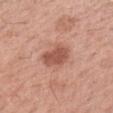Assessment: The lesion was tiled from a total-body skin photograph and was not biopsied. Acquisition and patient details: A roughly 15 mm field-of-view crop from a total-body skin photograph. The lesion-visualizer software estimated an area of roughly 8 mm² and two-axis asymmetry of about 0.25. Imaged with white-light lighting. A female subject, in their mid- to late 60s. From the arm.